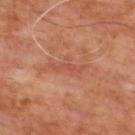Q: How was this image acquired?
A: total-body-photography crop, ~15 mm field of view
Q: What did automated image analysis measure?
A: an average lesion color of about L≈49 a*≈29 b*≈30 (CIELAB), roughly 6 lightness units darker than nearby skin, and a lesion-to-skin contrast of about 5 (normalized; higher = more distinct); a classifier nevus-likeness of about 0/100 and a detector confidence of about 85 out of 100 that the crop contains a lesion
Q: Who is the patient?
A: male, approximately 65 years of age
Q: How large is the lesion?
A: about 4.5 mm
Q: Lesion location?
A: the upper back